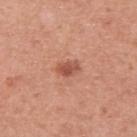Notes:
– automated metrics: an average lesion color of about L≈53 a*≈27 b*≈31 (CIELAB), a lesion–skin lightness drop of about 11, and a normalized border contrast of about 7.5; a color-variation rating of about 2.5/10; a classifier nevus-likeness of about 85/100 and lesion-presence confidence of about 100/100
– lesion size: ~2.5 mm (longest diameter)
– acquisition: 15 mm crop, total-body photography
– patient: male, in their 50s
– location: the upper back
– tile lighting: white-light illumination